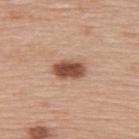{"biopsy_status": "not biopsied; imaged during a skin examination", "patient": {"sex": "female", "age_approx": 60}, "lesion_size": {"long_diameter_mm_approx": 4.0}, "automated_metrics": {"cielab_L": 51, "cielab_a": 22, "cielab_b": 29, "vs_skin_darker_L": 17.0, "vs_skin_contrast_norm": 11.0, "border_irregularity_0_10": 2.0, "color_variation_0_10": 4.0, "peripheral_color_asymmetry": 1.0}, "site": "upper back", "image": {"source": "total-body photography crop", "field_of_view_mm": 15}}